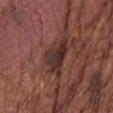No biopsy was performed on this lesion — it was imaged during a full skin examination and was not determined to be concerning.